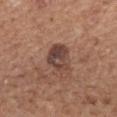The lesion was tiled from a total-body skin photograph and was not biopsied. Automated image analysis of the tile measured about 11 CIELAB-L* units darker than the surrounding skin and a normalized lesion–skin contrast near 9. The analysis additionally found a border-irregularity rating of about 2.5/10, internal color variation of about 6.5 on a 0–10 scale, and radial color variation of about 2. Captured under white-light illumination. Measured at roughly 4 mm in maximum diameter. Cropped from a total-body skin-imaging series; the visible field is about 15 mm. The patient is a male in their mid- to late 60s. From the mid back.